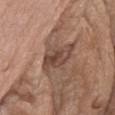TBP lesion metrics = a footprint of about 11 mm² and a shape eccentricity near 0.8; a within-lesion color-variation index near 5.5/10 and a peripheral color-asymmetry measure near 2 | body site = the chest | subject = male, roughly 75 years of age | imaging modality = ~15 mm tile from a whole-body skin photo | lesion diameter = ~5 mm (longest diameter).